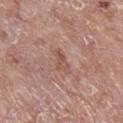{
  "biopsy_status": "not biopsied; imaged during a skin examination",
  "automated_metrics": {
    "area_mm2_approx": 4.0,
    "eccentricity": 0.85,
    "shape_asymmetry": 0.4,
    "vs_skin_darker_L": 7.0,
    "nevus_likeness_0_100": 0,
    "lesion_detection_confidence_0_100": 100
  },
  "image": {
    "source": "total-body photography crop",
    "field_of_view_mm": 15
  },
  "lighting": "white-light",
  "site": "right lower leg",
  "patient": {
    "sex": "female",
    "age_approx": 75
  }
}